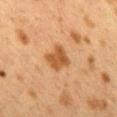Captured during whole-body skin photography for melanoma surveillance; the lesion was not biopsied. The lesion-visualizer software estimated an average lesion color of about L≈45 a*≈20 b*≈36 (CIELAB), about 10 CIELAB-L* units darker than the surrounding skin, and a lesion-to-skin contrast of about 8.5 (normalized; higher = more distinct). The software also gave a border-irregularity rating of about 2/10 and a peripheral color-asymmetry measure near 0.5. The analysis additionally found a nevus-likeness score of about 55/100 and lesion-presence confidence of about 100/100. A 15 mm close-up extracted from a 3D total-body photography capture. A female patient, aged approximately 40. The tile uses cross-polarized illumination. Longest diameter approximately 3 mm. The lesion is located on the mid back.